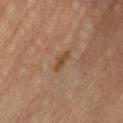This lesion was catalogued during total-body skin photography and was not selected for biopsy.
This is a cross-polarized tile.
Cropped from a total-body skin-imaging series; the visible field is about 15 mm.
A male patient aged 58–62.
Automated tile analysis of the lesion measured an automated nevus-likeness rating near 0 out of 100 and lesion-presence confidence of about 100/100.
The lesion is on the chest.
About 3 mm across.